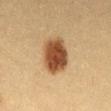Part of a total-body skin-imaging series; this lesion was reviewed on a skin check and was not flagged for biopsy. Located on the abdomen. Imaged with cross-polarized lighting. The patient is a female about 30 years old. A lesion tile, about 15 mm wide, cut from a 3D total-body photograph. The recorded lesion diameter is about 5 mm.